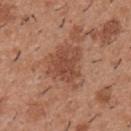Q: Was a biopsy performed?
A: total-body-photography surveillance lesion; no biopsy
Q: What is the imaging modality?
A: ~15 mm tile from a whole-body skin photo
Q: What are the patient's age and sex?
A: male, aged approximately 40
Q: What is the anatomic site?
A: the upper back
Q: How large is the lesion?
A: ~5.5 mm (longest diameter)